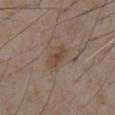automated metrics: a footprint of about 4.5 mm², a shape eccentricity near 0.85, and a shape-asymmetry score of about 0.3 (0 = symmetric); a border-irregularity index near 3.5/10, a color-variation rating of about 2.5/10, and radial color variation of about 0.5 | illumination: white-light | image source: 15 mm crop, total-body photography | location: the front of the torso | subject: male, in their mid-70s.